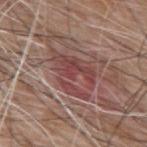Located on the upper back.
A region of skin cropped from a whole-body photographic capture, roughly 15 mm wide.
A male patient, roughly 60 years of age.